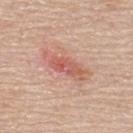Captured during whole-body skin photography for melanoma surveillance; the lesion was not biopsied. The patient is a male aged approximately 70. On the back. A roughly 15 mm field-of-view crop from a total-body skin photograph.